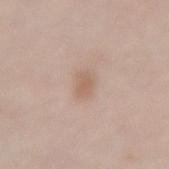Assessment: The lesion was tiled from a total-body skin photograph and was not biopsied. Acquisition and patient details: Imaged with white-light lighting. A roughly 15 mm field-of-view crop from a total-body skin photograph. Automated tile analysis of the lesion measured border irregularity of about 2.5 on a 0–10 scale. From the back. A female subject in their 50s. The lesion's longest dimension is about 3 mm.